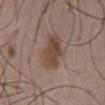workup: total-body-photography surveillance lesion; no biopsy
lesion diameter: ≈4.5 mm
automated metrics: a footprint of about 11 mm², an outline eccentricity of about 0.7 (0 = round, 1 = elongated), and a symmetry-axis asymmetry near 0.25; about 9 CIELAB-L* units darker than the surrounding skin; a color-variation rating of about 3.5/10 and peripheral color asymmetry of about 1; an automated nevus-likeness rating near 95 out of 100 and lesion-presence confidence of about 100/100
subject: male, approximately 40 years of age
illumination: white-light illumination
imaging modality: total-body-photography crop, ~15 mm field of view
location: the chest